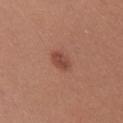Q: Is there a histopathology result?
A: total-body-photography surveillance lesion; no biopsy
Q: How was this image acquired?
A: 15 mm crop, total-body photography
Q: Lesion location?
A: the chest
Q: Illumination type?
A: white-light illumination
Q: Patient demographics?
A: female, aged around 25
Q: How large is the lesion?
A: ≈2.5 mm